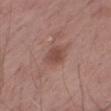Q: Is there a histopathology result?
A: no biopsy performed (imaged during a skin exam)